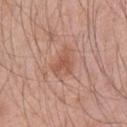No biopsy was performed on this lesion — it was imaged during a full skin examination and was not determined to be concerning. A male subject, about 45 years old. The lesion-visualizer software estimated border irregularity of about 6 on a 0–10 scale, a color-variation rating of about 1.5/10, and radial color variation of about 0.5. The software also gave a nevus-likeness score of about 5/100 and a detector confidence of about 100 out of 100 that the crop contains a lesion. A close-up tile cropped from a whole-body skin photograph, about 15 mm across. Captured under white-light illumination. From the left upper arm. The recorded lesion diameter is about 3.5 mm.